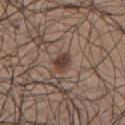Imaged during a routine full-body skin examination; the lesion was not biopsied and no histopathology is available. The lesion is on the front of the torso. A region of skin cropped from a whole-body photographic capture, roughly 15 mm wide. The lesion-visualizer software estimated an area of roughly 4 mm² and two-axis asymmetry of about 0.25. It also reported a border-irregularity index near 2/10, internal color variation of about 3 on a 0–10 scale, and peripheral color asymmetry of about 1. The analysis additionally found a nevus-likeness score of about 95/100 and a lesion-detection confidence of about 100/100. Measured at roughly 2.5 mm in maximum diameter. A male subject about 25 years old. This is a white-light tile.